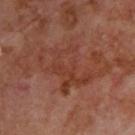Q: Was this lesion biopsied?
A: no biopsy performed (imaged during a skin exam)
Q: Where on the body is the lesion?
A: the upper back
Q: What is the imaging modality?
A: total-body-photography crop, ~15 mm field of view
Q: How large is the lesion?
A: ≈8 mm
Q: Illumination type?
A: cross-polarized illumination
Q: Who is the patient?
A: male, aged 68–72